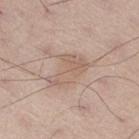Clinical impression:
This lesion was catalogued during total-body skin photography and was not selected for biopsy.
Acquisition and patient details:
Located on the right thigh. A lesion tile, about 15 mm wide, cut from a 3D total-body photograph. A male subject, about 60 years old.